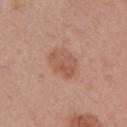Imaged during a routine full-body skin examination; the lesion was not biopsied and no histopathology is available.
Captured under white-light illumination.
The patient is a female aged around 55.
A lesion tile, about 15 mm wide, cut from a 3D total-body photograph.
Located on the arm.